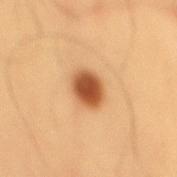Clinical impression: Part of a total-body skin-imaging series; this lesion was reviewed on a skin check and was not flagged for biopsy. Context: Cropped from a whole-body photographic skin survey; the tile spans about 15 mm. The lesion is located on the mid back. A male patient in their mid- to late 50s. The recorded lesion diameter is about 3.5 mm. Automated image analysis of the tile measured an area of roughly 7.5 mm², an outline eccentricity of about 0.55 (0 = round, 1 = elongated), and a symmetry-axis asymmetry near 0.15. It also reported a classifier nevus-likeness of about 100/100 and a lesion-detection confidence of about 100/100.